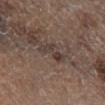- notes · catalogued during a skin exam; not biopsied
- lesion diameter · ~3.5 mm (longest diameter)
- imaging modality · ~15 mm crop, total-body skin-cancer survey
- tile lighting · cross-polarized
- body site · the right lower leg
- subject · male, about 65 years old